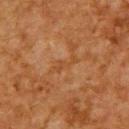Part of a total-body skin-imaging series; this lesion was reviewed on a skin check and was not flagged for biopsy.
On the upper back.
Cropped from a whole-body photographic skin survey; the tile spans about 15 mm.
The subject is a male approximately 65 years of age.